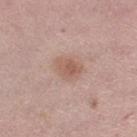This lesion was catalogued during total-body skin photography and was not selected for biopsy. A lesion tile, about 15 mm wide, cut from a 3D total-body photograph. The total-body-photography lesion software estimated an average lesion color of about L≈58 a*≈20 b*≈26 (CIELAB) and a lesion–skin lightness drop of about 8. The software also gave a border-irregularity rating of about 2/10, a color-variation rating of about 3/10, and peripheral color asymmetry of about 1. About 3 mm across. On the leg. The subject is a female aged 48 to 52. Captured under white-light illumination.